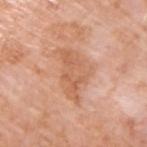This lesion was catalogued during total-body skin photography and was not selected for biopsy.
The tile uses white-light illumination.
A male patient approximately 60 years of age.
The lesion's longest dimension is about 6 mm.
On the right upper arm.
The lesion-visualizer software estimated a classifier nevus-likeness of about 0/100 and a detector confidence of about 100 out of 100 that the crop contains a lesion.
A roughly 15 mm field-of-view crop from a total-body skin photograph.